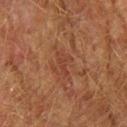| field | value |
|---|---|
| notes | imaged on a skin check; not biopsied |
| subject | male, roughly 75 years of age |
| site | the left forearm |
| acquisition | ~15 mm tile from a whole-body skin photo |
| tile lighting | cross-polarized illumination |
| size | ~3 mm (longest diameter) |